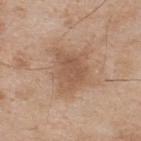Q: Where on the body is the lesion?
A: the upper back
Q: How was the tile lit?
A: white-light illumination
Q: What is the lesion's diameter?
A: ≈4.5 mm
Q: Automated lesion metrics?
A: a color-variation rating of about 2/10
Q: Who is the patient?
A: male, in their mid-70s
Q: What kind of image is this?
A: ~15 mm crop, total-body skin-cancer survey